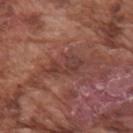Q: Was this lesion biopsied?
A: total-body-photography surveillance lesion; no biopsy
Q: What is the imaging modality?
A: ~15 mm crop, total-body skin-cancer survey
Q: What did automated image analysis measure?
A: a lesion area of about 6.5 mm², an eccentricity of roughly 0.85, and two-axis asymmetry of about 0.35; a mean CIELAB color near L≈39 a*≈22 b*≈24, a lesion–skin lightness drop of about 7, and a normalized border contrast of about 6.5; a classifier nevus-likeness of about 0/100
Q: What is the anatomic site?
A: the arm
Q: How large is the lesion?
A: about 4.5 mm
Q: How was the tile lit?
A: white-light
Q: What are the patient's age and sex?
A: male, about 75 years old